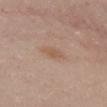Findings:
• site — the chest
• patient — female, aged 23–27
• image — ~15 mm crop, total-body skin-cancer survey
• lighting — white-light
• size — about 2.5 mm